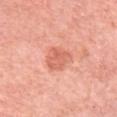biopsy status: total-body-photography surveillance lesion; no biopsy
anatomic site: the left upper arm
illumination: white-light
subject: female, about 55 years old
acquisition: 15 mm crop, total-body photography